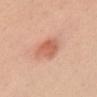<lesion>
<biopsy_status>not biopsied; imaged during a skin examination</biopsy_status>
<site>front of the torso</site>
<lesion_size>
  <long_diameter_mm_approx>3.5</long_diameter_mm_approx>
</lesion_size>
<image>
  <source>total-body photography crop</source>
  <field_of_view_mm>15</field_of_view_mm>
</image>
<lighting>white-light</lighting>
<patient>
  <sex>female</sex>
  <age_approx>55</age_approx>
</patient>
</lesion>